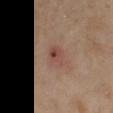Impression: No biopsy was performed on this lesion — it was imaged during a full skin examination and was not determined to be concerning. Background: A 15 mm close-up tile from a total-body photography series done for melanoma screening. The lesion-visualizer software estimated a lesion area of about 6 mm², an eccentricity of roughly 0.8, and a symmetry-axis asymmetry near 0.2. The software also gave about 7 CIELAB-L* units darker than the surrounding skin and a lesion-to-skin contrast of about 6 (normalized; higher = more distinct). The lesion's longest dimension is about 3.5 mm. Captured under cross-polarized illumination. A female patient, aged approximately 50. On the left upper arm.